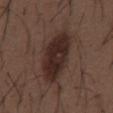This lesion was catalogued during total-body skin photography and was not selected for biopsy. A 15 mm close-up tile from a total-body photography series done for melanoma screening. Captured under white-light illumination. Automated image analysis of the tile measured a shape eccentricity near 0.85 and two-axis asymmetry of about 0.2. And it measured a mean CIELAB color near L≈27 a*≈16 b*≈19, roughly 10 lightness units darker than nearby skin, and a normalized border contrast of about 11. The software also gave a border-irregularity rating of about 3/10 and internal color variation of about 4 on a 0–10 scale. The analysis additionally found a nevus-likeness score of about 95/100 and lesion-presence confidence of about 100/100. The subject is a male in their 50s. From the back.